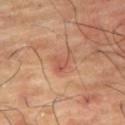Case summary:
* workup: total-body-photography surveillance lesion; no biopsy
* location: the right thigh
* image source: ~15 mm crop, total-body skin-cancer survey
* patient: male, aged approximately 65
* lesion diameter: about 2.5 mm